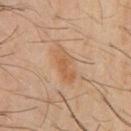| key | value |
|---|---|
| illumination | cross-polarized illumination |
| location | the chest |
| imaging modality | ~15 mm crop, total-body skin-cancer survey |
| patient | male, aged 58 to 62 |